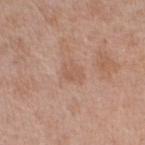workup = total-body-photography surveillance lesion; no biopsy
image = 15 mm crop, total-body photography
diameter = about 2.5 mm
body site = the right upper arm
illumination = white-light illumination
subject = male, aged 58–62